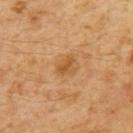image source: 15 mm crop, total-body photography
patient: male, roughly 60 years of age
site: the upper back